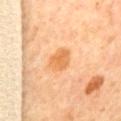Recorded during total-body skin imaging; not selected for excision or biopsy.
This image is a 15 mm lesion crop taken from a total-body photograph.
The lesion is on the back.
The total-body-photography lesion software estimated a footprint of about 5.5 mm², an eccentricity of roughly 0.75, and two-axis asymmetry of about 0.2. It also reported an average lesion color of about L≈67 a*≈26 b*≈46 (CIELAB), about 10 CIELAB-L* units darker than the surrounding skin, and a normalized lesion–skin contrast near 7.
A female subject, aged 63 to 67.
Imaged with cross-polarized lighting.